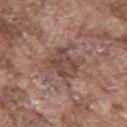Image and clinical context: A region of skin cropped from a whole-body photographic capture, roughly 15 mm wide. Located on the chest. Approximately 4 mm at its widest. The patient is a male in their mid- to late 70s. Imaged with white-light lighting.